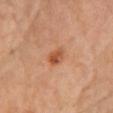This lesion was catalogued during total-body skin photography and was not selected for biopsy. A female subject roughly 60 years of age. Located on the chest. Cropped from a whole-body photographic skin survey; the tile spans about 15 mm.